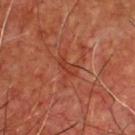No biopsy was performed on this lesion — it was imaged during a full skin examination and was not determined to be concerning. Measured at roughly 2.5 mm in maximum diameter. On the chest. A male patient, aged 63–67. Imaged with cross-polarized lighting. A 15 mm crop from a total-body photograph taken for skin-cancer surveillance.